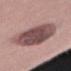This lesion was catalogued during total-body skin photography and was not selected for biopsy.
A 15 mm crop from a total-body photograph taken for skin-cancer surveillance.
Automated tile analysis of the lesion measured border irregularity of about 2.5 on a 0–10 scale, a color-variation rating of about 5/10, and a peripheral color-asymmetry measure near 1.5.
From the left thigh.
A female subject, about 40 years old.
The recorded lesion diameter is about 9 mm.